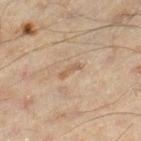Clinical summary:
A male patient roughly 45 years of age. A lesion tile, about 15 mm wide, cut from a 3D total-body photograph. This is a cross-polarized tile. Measured at roughly 2.5 mm in maximum diameter. From the right lower leg.